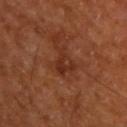Assessment: Imaged during a routine full-body skin examination; the lesion was not biopsied and no histopathology is available. Acquisition and patient details: This image is a 15 mm lesion crop taken from a total-body photograph. A male patient aged 63 to 67. Longest diameter approximately 3 mm. On the back. Captured under cross-polarized illumination.